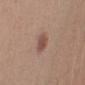The lesion was photographed on a routine skin check and not biopsied; there is no pathology result. A lesion tile, about 15 mm wide, cut from a 3D total-body photograph. A male subject aged around 55. On the right upper arm.